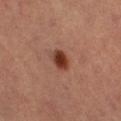biopsy status = catalogued during a skin exam; not biopsied | imaging modality = total-body-photography crop, ~15 mm field of view | subject = female, about 60 years old | lesion diameter = about 3 mm | anatomic site = the left thigh | tile lighting = cross-polarized illumination.